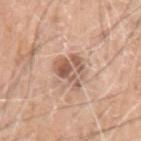notes — catalogued during a skin exam; not biopsied
tile lighting — white-light illumination
subject — male, aged 58–62
image-analysis metrics — a footprint of about 8.5 mm² and a symmetry-axis asymmetry near 0.4; roughly 12 lightness units darker than nearby skin and a lesion-to-skin contrast of about 8 (normalized; higher = more distinct)
body site — the left upper arm
acquisition — ~15 mm tile from a whole-body skin photo
size — ≈3.5 mm